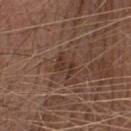Q: Was this lesion biopsied?
A: total-body-photography surveillance lesion; no biopsy